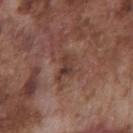biopsy status: total-body-photography surveillance lesion; no biopsy
site: the chest
image: ~15 mm tile from a whole-body skin photo
subject: male, aged around 75
lesion size: ~3 mm (longest diameter)
lighting: white-light illumination
automated metrics: a footprint of about 3.5 mm², an outline eccentricity of about 0.8 (0 = round, 1 = elongated), and a shape-asymmetry score of about 0.45 (0 = symmetric); a detector confidence of about 100 out of 100 that the crop contains a lesion